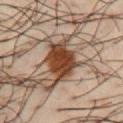follow-up — imaged on a skin check; not biopsied
subject — male, aged approximately 40
size — ≈5 mm
lighting — cross-polarized
imaging modality — ~15 mm tile from a whole-body skin photo
site — the chest